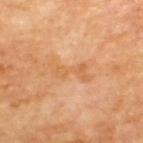No biopsy was performed on this lesion — it was imaged during a full skin examination and was not determined to be concerning.
A male patient, roughly 70 years of age.
The tile uses cross-polarized illumination.
The lesion's longest dimension is about 5 mm.
The lesion is on the back.
A lesion tile, about 15 mm wide, cut from a 3D total-body photograph.
The total-body-photography lesion software estimated a lesion area of about 6 mm². It also reported a border-irregularity index near 7/10 and radial color variation of about 1. It also reported a nevus-likeness score of about 0/100 and a lesion-detection confidence of about 100/100.